The patient is a male roughly 35 years of age; from the back; a 15 mm crop from a total-body photograph taken for skin-cancer surveillance: 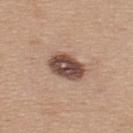The total-body-photography lesion software estimated lesion-presence confidence of about 100/100. Imaged with white-light lighting. The biopsy diagnosis was a benign skin lesion: dysplastic (Clark) nevus.